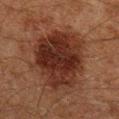This lesion was catalogued during total-body skin photography and was not selected for biopsy. Located on the right lower leg. The subject is a male about 60 years old. The lesion's longest dimension is about 7.5 mm. A 15 mm crop from a total-body photograph taken for skin-cancer surveillance. Automated tile analysis of the lesion measured an average lesion color of about L≈22 a*≈18 b*≈21 (CIELAB) and a normalized border contrast of about 11.5. And it measured a border-irregularity index near 2.5/10 and radial color variation of about 1.5. It also reported a nevus-likeness score of about 80/100 and a detector confidence of about 100 out of 100 that the crop contains a lesion. Imaged with cross-polarized lighting.